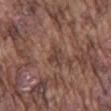The lesion was photographed on a routine skin check and not biopsied; there is no pathology result.